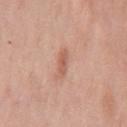{"biopsy_status": "not biopsied; imaged during a skin examination", "patient": {"sex": "male", "age_approx": 55}, "lesion_size": {"long_diameter_mm_approx": 3.5}, "image": {"source": "total-body photography crop", "field_of_view_mm": 15}, "site": "chest", "automated_metrics": {"area_mm2_approx": 3.5, "cielab_L": 59, "cielab_a": 24, "cielab_b": 29, "vs_skin_darker_L": 10.0, "vs_skin_contrast_norm": 6.5, "color_variation_0_10": 1.0, "peripheral_color_asymmetry": 0.5, "nevus_likeness_0_100": 80, "lesion_detection_confidence_0_100": 100}, "lighting": "white-light"}